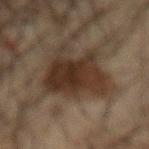Findings:
* biopsy status — no biopsy performed (imaged during a skin exam)
* image-analysis metrics — an area of roughly 26 mm², an eccentricity of roughly 0.5, and two-axis asymmetry of about 0.4; a classifier nevus-likeness of about 90/100
* anatomic site — the abdomen
* subject — male, roughly 65 years of age
* illumination — cross-polarized illumination
* image — total-body-photography crop, ~15 mm field of view
* lesion size — about 7 mm